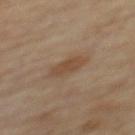Clinical impression: The lesion was photographed on a routine skin check and not biopsied; there is no pathology result. Background: The patient is a male aged 83 or over. The recorded lesion diameter is about 3.5 mm. A region of skin cropped from a whole-body photographic capture, roughly 15 mm wide. The lesion is on the upper back. Captured under cross-polarized illumination.